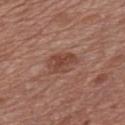biopsy status = catalogued during a skin exam; not biopsied | subject = male, aged 68–72 | acquisition = ~15 mm crop, total-body skin-cancer survey | body site = the front of the torso | size = about 4 mm | tile lighting = white-light.